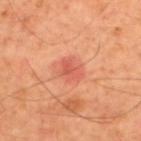Part of a total-body skin-imaging series; this lesion was reviewed on a skin check and was not flagged for biopsy.
The tile uses cross-polarized illumination.
An algorithmic analysis of the crop reported a footprint of about 5.5 mm², an eccentricity of roughly 0.55, and a shape-asymmetry score of about 0.3 (0 = symmetric). It also reported a border-irregularity rating of about 3/10, a color-variation rating of about 2.5/10, and radial color variation of about 1. The analysis additionally found a nevus-likeness score of about 20/100 and a lesion-detection confidence of about 100/100.
Measured at roughly 3 mm in maximum diameter.
Cropped from a whole-body photographic skin survey; the tile spans about 15 mm.
A male subject approximately 60 years of age.
The lesion is on the upper back.